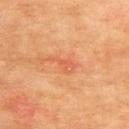Assessment: Imaged during a routine full-body skin examination; the lesion was not biopsied and no histopathology is available. Clinical summary: A male patient approximately 75 years of age. On the upper back. A roughly 15 mm field-of-view crop from a total-body skin photograph.